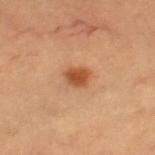Assessment:
The lesion was tiled from a total-body skin photograph and was not biopsied.
Clinical summary:
From the left leg. A lesion tile, about 15 mm wide, cut from a 3D total-body photograph. The subject is a female in their mid- to late 40s. The lesion's longest dimension is about 2.5 mm. The total-body-photography lesion software estimated a border-irregularity rating of about 2/10, a within-lesion color-variation index near 3/10, and peripheral color asymmetry of about 1. The analysis additionally found a classifier nevus-likeness of about 100/100 and lesion-presence confidence of about 100/100. The tile uses cross-polarized illumination.